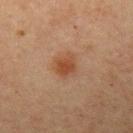Q: Is there a histopathology result?
A: imaged on a skin check; not biopsied
Q: Who is the patient?
A: male, aged 58–62
Q: How was this image acquired?
A: 15 mm crop, total-body photography
Q: What lighting was used for the tile?
A: cross-polarized
Q: Lesion location?
A: the left upper arm